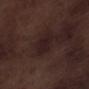Clinical impression: No biopsy was performed on this lesion — it was imaged during a full skin examination and was not determined to be concerning. Image and clinical context: A male patient, aged around 70. A 15 mm crop from a total-body photograph taken for skin-cancer surveillance. This is a white-light tile. The lesion-visualizer software estimated a footprint of about 7 mm², an eccentricity of roughly 0.75, and two-axis asymmetry of about 0.3. The software also gave a mean CIELAB color near L≈19 a*≈15 b*≈14 and a normalized border contrast of about 7.5. It also reported a within-lesion color-variation index near 2/10 and peripheral color asymmetry of about 1. The software also gave a classifier nevus-likeness of about 0/100. Approximately 3.5 mm at its widest. The lesion is on the left lower leg.